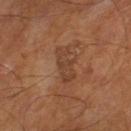{"biopsy_status": "not biopsied; imaged during a skin examination", "lighting": "cross-polarized", "automated_metrics": {"area_mm2_approx": 7.0, "eccentricity": 0.9, "shape_asymmetry": 0.3, "cielab_L": 39, "cielab_a": 20, "cielab_b": 30, "vs_skin_darker_L": 7.0, "vs_skin_contrast_norm": 6.0, "border_irregularity_0_10": 5.0}, "image": {"source": "total-body photography crop", "field_of_view_mm": 15}, "lesion_size": {"long_diameter_mm_approx": 4.5}, "patient": {"sex": "male", "age_approx": 65}, "site": "leg"}